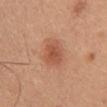<record>
  <biopsy_status>not biopsied; imaged during a skin examination</biopsy_status>
  <lighting>white-light</lighting>
  <patient>
    <sex>female</sex>
    <age_approx>45</age_approx>
  </patient>
  <lesion_size>
    <long_diameter_mm_approx>4.0</long_diameter_mm_approx>
  </lesion_size>
  <image>
    <source>total-body photography crop</source>
    <field_of_view_mm>15</field_of_view_mm>
  </image>
  <site>chest</site>
</record>